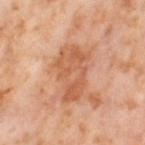Findings:
* follow-up: no biopsy performed (imaged during a skin exam)
* location: the leg
* size: about 6.5 mm
* subject: female, aged 53 to 57
* lighting: cross-polarized illumination
* TBP lesion metrics: a mean CIELAB color near L≈60 a*≈26 b*≈36, about 10 CIELAB-L* units darker than the surrounding skin, and a normalized lesion–skin contrast near 6.5; a border-irregularity index near 3.5/10 and a within-lesion color-variation index near 4.5/10; an automated nevus-likeness rating near 0 out of 100 and a lesion-detection confidence of about 100/100
* acquisition: ~15 mm crop, total-body skin-cancer survey